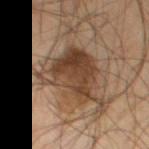Imaged during a routine full-body skin examination; the lesion was not biopsied and no histopathology is available. A 15 mm close-up extracted from a 3D total-body photography capture. A male patient approximately 55 years of age. The lesion's longest dimension is about 7.5 mm. Located on the left thigh. Automated tile analysis of the lesion measured a lesion area of about 22 mm², an eccentricity of roughly 0.45, and a symmetry-axis asymmetry near 0.45. The software also gave a mean CIELAB color near L≈38 a*≈16 b*≈27, roughly 12 lightness units darker than nearby skin, and a normalized border contrast of about 10. It also reported a within-lesion color-variation index near 6/10 and peripheral color asymmetry of about 2. The analysis additionally found an automated nevus-likeness rating near 40 out of 100 and a lesion-detection confidence of about 100/100. The tile uses cross-polarized illumination.